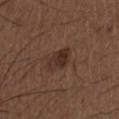  biopsy_status: not biopsied; imaged during a skin examination
  lighting: white-light
  site: chest
  image:
    source: total-body photography crop
    field_of_view_mm: 15
  lesion_size:
    long_diameter_mm_approx: 3.5
  patient:
    sex: male
    age_approx: 50